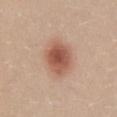Q: Was a biopsy performed?
A: total-body-photography surveillance lesion; no biopsy
Q: Where on the body is the lesion?
A: the lower back
Q: How was the tile lit?
A: white-light
Q: How was this image acquired?
A: 15 mm crop, total-body photography
Q: What are the patient's age and sex?
A: female, aged around 20